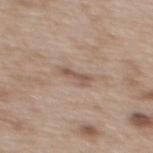notes=catalogued during a skin exam; not biopsied
location=the upper back
subject=female, about 40 years old
image-analysis metrics=a border-irregularity rating of about 5/10, a within-lesion color-variation index near 0/10, and radial color variation of about 0; an automated nevus-likeness rating near 0 out of 100
image source=15 mm crop, total-body photography
size=≈3 mm
lighting=white-light